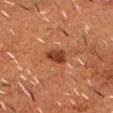Case summary:
• biopsy status: catalogued during a skin exam; not biopsied
• lesion diameter: ≈2.5 mm
• subject: male, approximately 55 years of age
• illumination: cross-polarized
• automated lesion analysis: a mean CIELAB color near L≈38 a*≈27 b*≈33, about 12 CIELAB-L* units darker than the surrounding skin, and a normalized lesion–skin contrast near 10
• imaging modality: ~15 mm tile from a whole-body skin photo
• location: the head or neck